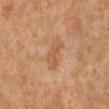The lesion was tiled from a total-body skin photograph and was not biopsied.
A 15 mm close-up tile from a total-body photography series done for melanoma screening.
The lesion's longest dimension is about 4 mm.
A female subject aged 63–67.
Imaged with cross-polarized lighting.
The lesion is located on the right lower leg.